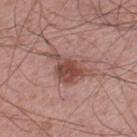A 15 mm crop from a total-body photograph taken for skin-cancer surveillance. Captured under white-light illumination. The recorded lesion diameter is about 6 mm. An algorithmic analysis of the crop reported a footprint of about 11 mm², an eccentricity of roughly 0.8, and two-axis asymmetry of about 0.45. The analysis additionally found a mean CIELAB color near L≈48 a*≈22 b*≈24 and about 11 CIELAB-L* units darker than the surrounding skin. And it measured a border-irregularity index near 6/10 and peripheral color asymmetry of about 1. And it measured a detector confidence of about 100 out of 100 that the crop contains a lesion. The subject is a male approximately 55 years of age. On the leg.